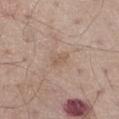Impression: The lesion was photographed on a routine skin check and not biopsied; there is no pathology result. Image and clinical context: Captured under white-light illumination. The lesion is located on the left thigh. This image is a 15 mm lesion crop taken from a total-body photograph. About 2.5 mm across. The subject is a male roughly 65 years of age.